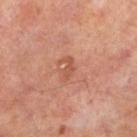No biopsy was performed on this lesion — it was imaged during a full skin examination and was not determined to be concerning.
The lesion is located on the left lower leg.
Imaged with cross-polarized lighting.
A 15 mm crop from a total-body photograph taken for skin-cancer surveillance.
The lesion-visualizer software estimated an outline eccentricity of about 0.85 (0 = round, 1 = elongated). And it measured a lesion color around L≈51 a*≈26 b*≈32 in CIELAB, a lesion–skin lightness drop of about 7, and a lesion-to-skin contrast of about 5.5 (normalized; higher = more distinct). And it measured a color-variation rating of about 0.5/10 and radial color variation of about 0. It also reported a classifier nevus-likeness of about 0/100 and lesion-presence confidence of about 100/100.
A male patient aged 63–67.